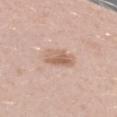Clinical impression: The lesion was photographed on a routine skin check and not biopsied; there is no pathology result. Context: The recorded lesion diameter is about 3.5 mm. The lesion is located on the left forearm. A region of skin cropped from a whole-body photographic capture, roughly 15 mm wide. Imaged with white-light lighting. An algorithmic analysis of the crop reported a lesion area of about 7.5 mm², a shape eccentricity near 0.6, and a symmetry-axis asymmetry near 0.2. A female subject, aged 18–22.